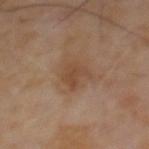Imaged during a routine full-body skin examination; the lesion was not biopsied and no histopathology is available. The patient is a male aged approximately 65. An algorithmic analysis of the crop reported a footprint of about 6.5 mm². The software also gave a lesion color around L≈44 a*≈17 b*≈29 in CIELAB and a normalized border contrast of about 6. And it measured a border-irregularity index near 5/10, a color-variation rating of about 2/10, and radial color variation of about 0.5. Imaged with cross-polarized lighting. The recorded lesion diameter is about 3.5 mm. A 15 mm crop from a total-body photograph taken for skin-cancer surveillance.